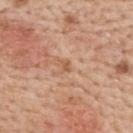| key | value |
|---|---|
| notes | total-body-photography surveillance lesion; no biopsy |
| automated metrics | an area of roughly 1 mm² and two-axis asymmetry of about 0.55; a mean CIELAB color near L≈57 a*≈22 b*≈35, a lesion–skin lightness drop of about 8, and a lesion-to-skin contrast of about 6.5 (normalized; higher = more distinct); a color-variation rating of about 0/10 and radial color variation of about 0; a nevus-likeness score of about 0/100 and a detector confidence of about 100 out of 100 that the crop contains a lesion |
| location | the upper back |
| lesion size | about 1 mm |
| image | ~15 mm tile from a whole-body skin photo |
| subject | female, aged approximately 50 |